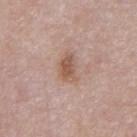The lesion was tiled from a total-body skin photograph and was not biopsied.
Automated tile analysis of the lesion measured a nevus-likeness score of about 55/100 and lesion-presence confidence of about 100/100.
A male subject, aged 53 to 57.
The recorded lesion diameter is about 3.5 mm.
A region of skin cropped from a whole-body photographic capture, roughly 15 mm wide.
The tile uses white-light illumination.
From the chest.